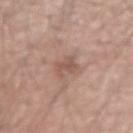Image and clinical context: This is a white-light tile. A roughly 15 mm field-of-view crop from a total-body skin photograph. Approximately 2.5 mm at its widest. The patient is a male in their mid- to late 50s. On the left forearm. The total-body-photography lesion software estimated a shape eccentricity near 0.5 and two-axis asymmetry of about 0.4. And it measured a border-irregularity index near 5/10, internal color variation of about 1.5 on a 0–10 scale, and peripheral color asymmetry of about 0.5. The analysis additionally found lesion-presence confidence of about 100/100.